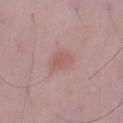Case summary:
• workup — catalogued during a skin exam; not biopsied
• location — the left upper arm
• lesion size — ≈3 mm
• lighting — white-light illumination
• patient — male, approximately 50 years of age
• automated lesion analysis — an area of roughly 4.5 mm², an outline eccentricity of about 0.8 (0 = round, 1 = elongated), and two-axis asymmetry of about 0.2; an average lesion color of about L≈56 a*≈21 b*≈23 (CIELAB) and roughly 7 lightness units darker than nearby skin; border irregularity of about 2 on a 0–10 scale and internal color variation of about 1.5 on a 0–10 scale; a nevus-likeness score of about 25/100 and lesion-presence confidence of about 100/100
• image source — 15 mm crop, total-body photography